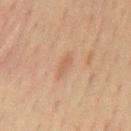Assessment:
Captured during whole-body skin photography for melanoma surveillance; the lesion was not biopsied.
Background:
A region of skin cropped from a whole-body photographic capture, roughly 15 mm wide. Imaged with cross-polarized lighting. A male subject roughly 70 years of age. From the chest. The lesion's longest dimension is about 3.5 mm.